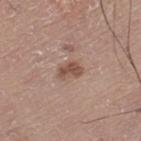This lesion was catalogued during total-body skin photography and was not selected for biopsy.
Measured at roughly 3 mm in maximum diameter.
Imaged with white-light lighting.
The lesion-visualizer software estimated a lesion color around L≈50 a*≈20 b*≈26 in CIELAB and a lesion–skin lightness drop of about 11.
The patient is a male aged 58–62.
A lesion tile, about 15 mm wide, cut from a 3D total-body photograph.
On the left thigh.